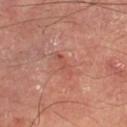Part of a total-body skin-imaging series; this lesion was reviewed on a skin check and was not flagged for biopsy.
A roughly 15 mm field-of-view crop from a total-body skin photograph.
The patient is a male aged 63–67.
From the left lower leg.